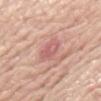Impression: The lesion was photographed on a routine skin check and not biopsied; there is no pathology result. Acquisition and patient details: Captured under white-light illumination. The total-body-photography lesion software estimated a border-irregularity index near 2/10, a within-lesion color-variation index near 3.5/10, and a peripheral color-asymmetry measure near 1.5. A 15 mm crop from a total-body photograph taken for skin-cancer surveillance. Approximately 3 mm at its widest. The lesion is located on the abdomen. A female patient, aged 68–72.